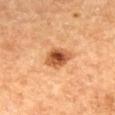Impression:
Part of a total-body skin-imaging series; this lesion was reviewed on a skin check and was not flagged for biopsy.
Acquisition and patient details:
A female subject approximately 60 years of age. A 15 mm crop from a total-body photograph taken for skin-cancer surveillance. Measured at roughly 4 mm in maximum diameter. Captured under cross-polarized illumination. On the mid back. Automated tile analysis of the lesion measured a footprint of about 7 mm², a shape eccentricity near 0.8, and a symmetry-axis asymmetry near 0.2.